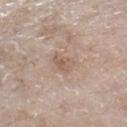<tbp_lesion>
  <biopsy_status>not biopsied; imaged during a skin examination</biopsy_status>
  <patient>
    <sex>female</sex>
    <age_approx>75</age_approx>
  </patient>
  <automated_metrics>
    <cielab_L>58</cielab_L>
    <cielab_a>15</cielab_a>
    <cielab_b>26</cielab_b>
    <vs_skin_darker_L>7.0</vs_skin_darker_L>
    <nevus_likeness_0_100>0</nevus_likeness_0_100>
    <lesion_detection_confidence_0_100>100</lesion_detection_confidence_0_100>
  </automated_metrics>
  <lesion_size>
    <long_diameter_mm_approx>3.0</long_diameter_mm_approx>
  </lesion_size>
  <lighting>white-light</lighting>
  <image>
    <source>total-body photography crop</source>
    <field_of_view_mm>15</field_of_view_mm>
  </image>
  <site>right lower leg</site>
</tbp_lesion>